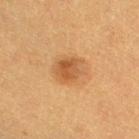Impression: Captured during whole-body skin photography for melanoma surveillance; the lesion was not biopsied. Acquisition and patient details: An algorithmic analysis of the crop reported a shape-asymmetry score of about 0.2 (0 = symmetric). The analysis additionally found a lesion color around L≈45 a*≈19 b*≈34 in CIELAB, roughly 8 lightness units darker than nearby skin, and a lesion-to-skin contrast of about 6.5 (normalized; higher = more distinct). From the right thigh. This is a cross-polarized tile. A female subject about 40 years old. A region of skin cropped from a whole-body photographic capture, roughly 15 mm wide. Measured at roughly 4 mm in maximum diameter.